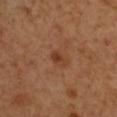notes: catalogued during a skin exam; not biopsied | diameter: ~2.5 mm (longest diameter) | illumination: cross-polarized | subject: male, aged approximately 50 | site: the left upper arm | image: ~15 mm crop, total-body skin-cancer survey.